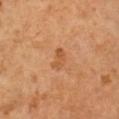workup: catalogued during a skin exam; not biopsied | anatomic site: the right arm | image source: ~15 mm tile from a whole-body skin photo | subject: male, roughly 60 years of age.